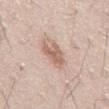The lesion-visualizer software estimated an area of roughly 6.5 mm², an outline eccentricity of about 0.6 (0 = round, 1 = elongated), and a shape-asymmetry score of about 0.3 (0 = symmetric). The software also gave about 11 CIELAB-L* units darker than the surrounding skin and a lesion-to-skin contrast of about 7 (normalized; higher = more distinct). It also reported an automated nevus-likeness rating near 65 out of 100 and a lesion-detection confidence of about 100/100. Located on the abdomen. A 15 mm close-up extracted from a 3D total-body photography capture. Approximately 3 mm at its widest. A male subject, aged 43 to 47. Captured under white-light illumination.